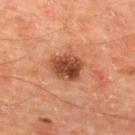Captured during whole-body skin photography for melanoma surveillance; the lesion was not biopsied. On the back. Cropped from a whole-body photographic skin survey; the tile spans about 15 mm. A male subject roughly 65 years of age. Measured at roughly 4 mm in maximum diameter. Imaged with cross-polarized lighting.